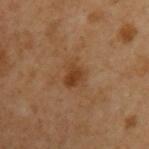Measured at roughly 3.5 mm in maximum diameter.
The lesion is located on the arm.
A roughly 15 mm field-of-view crop from a total-body skin photograph.
The lesion-visualizer software estimated a lesion area of about 6 mm² and a shape-asymmetry score of about 0.3 (0 = symmetric). It also reported a lesion color around L≈41 a*≈20 b*≈34 in CIELAB. The software also gave a border-irregularity rating of about 3/10. The software also gave a nevus-likeness score of about 65/100 and lesion-presence confidence of about 100/100.
The patient is a male roughly 50 years of age.
This is a cross-polarized tile.